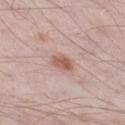Clinical impression: Captured during whole-body skin photography for melanoma surveillance; the lesion was not biopsied. Acquisition and patient details: A 15 mm close-up tile from a total-body photography series done for melanoma screening. Located on the left thigh. A male subject aged approximately 55.